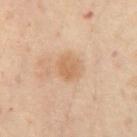This lesion was catalogued during total-body skin photography and was not selected for biopsy.
The patient is a female aged around 55.
The lesion's longest dimension is about 2.5 mm.
A lesion tile, about 15 mm wide, cut from a 3D total-body photograph.
On the abdomen.